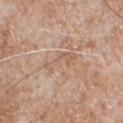{"biopsy_status": "not biopsied; imaged during a skin examination", "patient": {"sex": "male", "age_approx": 65}, "lighting": "white-light", "lesion_size": {"long_diameter_mm_approx": 4.5}, "site": "chest", "image": {"source": "total-body photography crop", "field_of_view_mm": 15}}